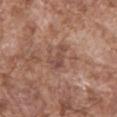Findings:
* follow-up: catalogued during a skin exam; not biopsied
* subject: male, aged approximately 75
* lighting: white-light
* automated lesion analysis: an area of roughly 4.5 mm², an eccentricity of roughly 0.9, and two-axis asymmetry of about 0.45; a mean CIELAB color near L≈48 a*≈20 b*≈25, a lesion–skin lightness drop of about 8, and a normalized border contrast of about 6.5; a classifier nevus-likeness of about 0/100 and a lesion-detection confidence of about 100/100
* lesion diameter: ~3.5 mm (longest diameter)
* anatomic site: the abdomen
* image source: total-body-photography crop, ~15 mm field of view